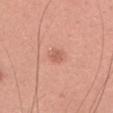The lesion was photographed on a routine skin check and not biopsied; there is no pathology result.
A 15 mm close-up extracted from a 3D total-body photography capture.
A female patient, aged approximately 35.
Automated image analysis of the tile measured a lesion color around L≈59 a*≈26 b*≈30 in CIELAB and a normalized lesion–skin contrast near 5.5. The software also gave internal color variation of about 1 on a 0–10 scale.
The lesion's longest dimension is about 2 mm.
Located on the head or neck.
Captured under white-light illumination.